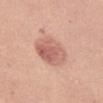Findings:
* lighting: white-light
* imaging modality: ~15 mm tile from a whole-body skin photo
* site: the left thigh
* subject: female, aged 43–47
* TBP lesion metrics: a mean CIELAB color near L≈61 a*≈25 b*≈27 and a lesion-to-skin contrast of about 7 (normalized; higher = more distinct); a border-irregularity index near 1/10 and internal color variation of about 5.5 on a 0–10 scale; a nevus-likeness score of about 80/100 and a lesion-detection confidence of about 100/100